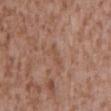biopsy status = catalogued during a skin exam; not biopsied | tile lighting = white-light illumination | image = ~15 mm crop, total-body skin-cancer survey | body site = the mid back | patient = male, in their mid-40s | lesion diameter = ≈2.5 mm | image-analysis metrics = a footprint of about 3 mm², a shape eccentricity near 0.85, and two-axis asymmetry of about 0.35; a lesion–skin lightness drop of about 5 and a normalized lesion–skin contrast near 4.5; internal color variation of about 0 on a 0–10 scale and peripheral color asymmetry of about 0.